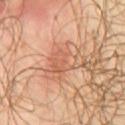diameter=≈5 mm; image=total-body-photography crop, ~15 mm field of view; anatomic site=the front of the torso; TBP lesion metrics=a footprint of about 12 mm², a shape eccentricity near 0.85, and a symmetry-axis asymmetry near 0.25; patient=male, aged 53 to 57; lighting=cross-polarized.